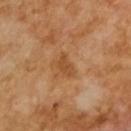biopsy status — catalogued during a skin exam; not biopsied | image-analysis metrics — a mean CIELAB color near L≈47 a*≈22 b*≈38, about 8 CIELAB-L* units darker than the surrounding skin, and a normalized lesion–skin contrast near 6.5; an automated nevus-likeness rating near 0 out of 100 and a lesion-detection confidence of about 100/100 | patient — male, aged around 65 | lesion size — about 3 mm | image source — total-body-photography crop, ~15 mm field of view | tile lighting — cross-polarized illumination.